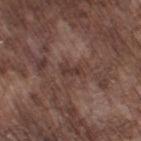<lesion>
<biopsy_status>not biopsied; imaged during a skin examination</biopsy_status>
<site>leg</site>
<patient>
  <sex>male</sex>
  <age_approx>75</age_approx>
</patient>
<image>
  <source>total-body photography crop</source>
  <field_of_view_mm>15</field_of_view_mm>
</image>
</lesion>